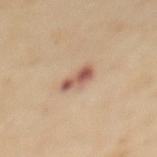Case summary:
* notes — catalogued during a skin exam; not biopsied
* size — ≈3.5 mm
* image source — ~15 mm crop, total-body skin-cancer survey
* anatomic site — the upper back
* image-analysis metrics — a mean CIELAB color near L≈56 a*≈21 b*≈28, about 13 CIELAB-L* units darker than the surrounding skin, and a normalized border contrast of about 9; border irregularity of about 3.5 on a 0–10 scale, a within-lesion color-variation index near 3.5/10, and peripheral color asymmetry of about 1
* patient — female, roughly 45 years of age